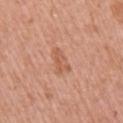follow-up: total-body-photography surveillance lesion; no biopsy | patient: female, aged 38 to 42 | location: the right upper arm | acquisition: ~15 mm tile from a whole-body skin photo | TBP lesion metrics: an average lesion color of about L≈58 a*≈25 b*≈33 (CIELAB), roughly 8 lightness units darker than nearby skin, and a lesion-to-skin contrast of about 5.5 (normalized; higher = more distinct); an automated nevus-likeness rating near 0 out of 100 and lesion-presence confidence of about 100/100 | lighting: white-light | size: ~3 mm (longest diameter).